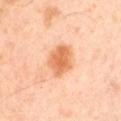  biopsy_status: not biopsied; imaged during a skin examination
  patient:
    sex: male
    age_approx: 45
  image:
    source: total-body photography crop
    field_of_view_mm: 15
  lighting: cross-polarized
  lesion_size:
    long_diameter_mm_approx: 4.0
  site: left upper arm